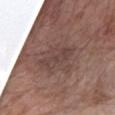follow-up: imaged on a skin check; not biopsied
image-analysis metrics: an area of roughly 9 mm², an outline eccentricity of about 0.9 (0 = round, 1 = elongated), and a symmetry-axis asymmetry near 0.3; a lesion color around L≈42 a*≈17 b*≈20 in CIELAB and a lesion–skin lightness drop of about 7
subject: male, approximately 60 years of age
image source: 15 mm crop, total-body photography
diameter: ~5.5 mm (longest diameter)
anatomic site: the arm